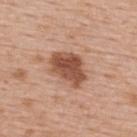Assessment:
No biopsy was performed on this lesion — it was imaged during a full skin examination and was not determined to be concerning.
Clinical summary:
The subject is a female aged around 65. Measured at roughly 5 mm in maximum diameter. Imaged with white-light lighting. A close-up tile cropped from a whole-body skin photograph, about 15 mm across. Located on the upper back.